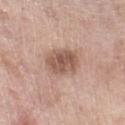Clinical impression: Imaged during a routine full-body skin examination; the lesion was not biopsied and no histopathology is available. Image and clinical context: A roughly 15 mm field-of-view crop from a total-body skin photograph. The lesion is located on the right lower leg. The recorded lesion diameter is about 4 mm. The tile uses white-light illumination. A female subject, aged approximately 55. An algorithmic analysis of the crop reported a lesion–skin lightness drop of about 12 and a normalized lesion–skin contrast near 8. And it measured a peripheral color-asymmetry measure near 1.5. And it measured an automated nevus-likeness rating near 30 out of 100 and a detector confidence of about 100 out of 100 that the crop contains a lesion.